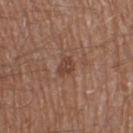Impression: No biopsy was performed on this lesion — it was imaged during a full skin examination and was not determined to be concerning. Context: Captured under white-light illumination. The lesion is located on the leg. A male patient, aged 63 to 67. Automated tile analysis of the lesion measured a lesion color around L≈42 a*≈21 b*≈27 in CIELAB, a lesion–skin lightness drop of about 8, and a normalized lesion–skin contrast near 7. It also reported border irregularity of about 3 on a 0–10 scale, internal color variation of about 1.5 on a 0–10 scale, and radial color variation of about 0.5. The software also gave a nevus-likeness score of about 0/100 and lesion-presence confidence of about 100/100. A 15 mm close-up extracted from a 3D total-body photography capture.